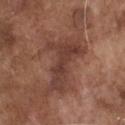The lesion is on the chest.
The lesion-visualizer software estimated an area of roughly 19 mm², a shape eccentricity near 0.85, and a shape-asymmetry score of about 0.55 (0 = symmetric). The software also gave internal color variation of about 3.5 on a 0–10 scale and peripheral color asymmetry of about 1.
The tile uses white-light illumination.
The subject is a male approximately 75 years of age.
A 15 mm close-up tile from a total-body photography series done for melanoma screening.
Approximately 7 mm at its widest.